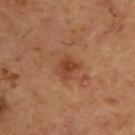Impression: Captured during whole-body skin photography for melanoma surveillance; the lesion was not biopsied. Context: A male patient aged around 55. A 15 mm close-up tile from a total-body photography series done for melanoma screening. The lesion is located on the upper back.